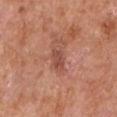This lesion was catalogued during total-body skin photography and was not selected for biopsy. About 3.5 mm across. A roughly 15 mm field-of-view crop from a total-body skin photograph. A male patient, aged 63 to 67. The lesion is located on the left upper arm. Imaged with white-light lighting.